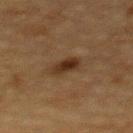<case>
  <site>upper back</site>
  <automated_metrics>
    <area_mm2_approx>5.0</area_mm2_approx>
    <shape_asymmetry>0.2</shape_asymmetry>
  </automated_metrics>
  <lesion_size>
    <long_diameter_mm_approx>3.0</long_diameter_mm_approx>
  </lesion_size>
  <lighting>cross-polarized</lighting>
  <patient>
    <sex>female</sex>
    <age_approx>55</age_approx>
  </patient>
  <image>
    <source>total-body photography crop</source>
    <field_of_view_mm>15</field_of_view_mm>
  </image>
</case>